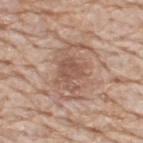Captured during whole-body skin photography for melanoma surveillance; the lesion was not biopsied. Imaged with white-light lighting. The subject is a male approximately 80 years of age. A close-up tile cropped from a whole-body skin photograph, about 15 mm across. The lesion is on the upper back. About 6.5 mm across. The total-body-photography lesion software estimated an area of roughly 20 mm², a shape eccentricity near 0.8, and two-axis asymmetry of about 0.3. It also reported an average lesion color of about L≈56 a*≈19 b*≈27 (CIELAB), a lesion–skin lightness drop of about 9, and a lesion-to-skin contrast of about 6 (normalized; higher = more distinct). The analysis additionally found a nevus-likeness score of about 30/100 and a detector confidence of about 65 out of 100 that the crop contains a lesion.